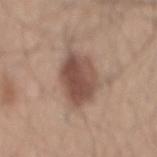biopsy status=imaged on a skin check; not biopsied | subject=male, in their mid-40s | imaging modality=~15 mm tile from a whole-body skin photo | site=the back | automated metrics=about 13 CIELAB-L* units darker than the surrounding skin and a normalized lesion–skin contrast near 9.5 | lighting=white-light illumination.